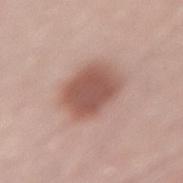The lesion was photographed on a routine skin check and not biopsied; there is no pathology result.
A 15 mm close-up extracted from a 3D total-body photography capture.
The recorded lesion diameter is about 5.5 mm.
On the lower back.
A female patient roughly 40 years of age.
Automated image analysis of the tile measured a lesion area of about 17 mm² and an eccentricity of roughly 0.65. It also reported a lesion color around L≈54 a*≈22 b*≈25 in CIELAB, roughly 13 lightness units darker than nearby skin, and a lesion-to-skin contrast of about 8.5 (normalized; higher = more distinct). It also reported a within-lesion color-variation index near 3/10 and a peripheral color-asymmetry measure near 1. It also reported a classifier nevus-likeness of about 90/100 and lesion-presence confidence of about 100/100.